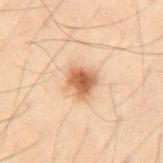Clinical summary:
The lesion is on the back. A roughly 15 mm field-of-view crop from a total-body skin photograph. Measured at roughly 3.5 mm in maximum diameter. The patient is a male aged 28–32. Captured under cross-polarized illumination.